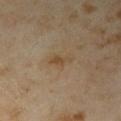Q: Is there a histopathology result?
A: imaged on a skin check; not biopsied
Q: What is the anatomic site?
A: the right upper arm
Q: Lesion size?
A: about 3 mm
Q: Automated lesion metrics?
A: a footprint of about 3.5 mm², an outline eccentricity of about 0.9 (0 = round, 1 = elongated), and a shape-asymmetry score of about 0.35 (0 = symmetric); a classifier nevus-likeness of about 10/100 and a detector confidence of about 100 out of 100 that the crop contains a lesion
Q: What is the imaging modality?
A: 15 mm crop, total-body photography
Q: Patient demographics?
A: female, about 35 years old
Q: Illumination type?
A: cross-polarized illumination